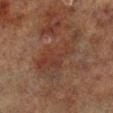Assessment: The lesion was tiled from a total-body skin photograph and was not biopsied. Image and clinical context: Automated tile analysis of the lesion measured a lesion color around L≈30 a*≈17 b*≈23 in CIELAB, about 5 CIELAB-L* units darker than the surrounding skin, and a lesion-to-skin contrast of about 5.5 (normalized; higher = more distinct). The software also gave a border-irregularity index near 6.5/10, internal color variation of about 4 on a 0–10 scale, and radial color variation of about 1.5. The software also gave lesion-presence confidence of about 100/100. Measured at roughly 7 mm in maximum diameter. The tile uses cross-polarized illumination. The lesion is located on the right lower leg. A male subject, roughly 70 years of age. Cropped from a whole-body photographic skin survey; the tile spans about 15 mm.